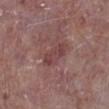Case summary:
– notes · total-body-photography surveillance lesion; no biopsy
– subject · male, in their mid-70s
– size · ≈4 mm
– automated metrics · a lesion area of about 6.5 mm², a shape eccentricity near 0.9, and a shape-asymmetry score of about 0.25 (0 = symmetric); a lesion color around L≈42 a*≈23 b*≈19 in CIELAB, a lesion–skin lightness drop of about 7, and a normalized lesion–skin contrast near 6
– image source · total-body-photography crop, ~15 mm field of view
– body site · the left lower leg